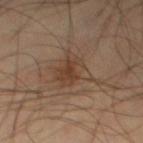Assessment: Recorded during total-body skin imaging; not selected for excision or biopsy. Background: Cropped from a total-body skin-imaging series; the visible field is about 15 mm. Located on the left thigh. A male patient, aged approximately 45.